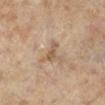* biopsy status: no biopsy performed (imaged during a skin exam)
* body site: the right lower leg
* image: ~15 mm crop, total-body skin-cancer survey
* lesion size: ~2.5 mm (longest diameter)
* subject: female, aged approximately 60